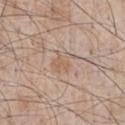Clinical impression:
This lesion was catalogued during total-body skin photography and was not selected for biopsy.
Background:
Approximately 3 mm at its widest. The subject is a male aged 63–67. The lesion is on the chest. A roughly 15 mm field-of-view crop from a total-body skin photograph. Captured under white-light illumination.